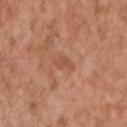Notes:
• follow-up — imaged on a skin check; not biopsied
• site — the upper back
• subject — male, aged around 65
• automated lesion analysis — border irregularity of about 3.5 on a 0–10 scale, a color-variation rating of about 1/10, and peripheral color asymmetry of about 0.5; a nevus-likeness score of about 0/100
• tile lighting — white-light
• lesion diameter — ≈3 mm
• image source — 15 mm crop, total-body photography